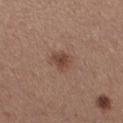Captured during whole-body skin photography for melanoma surveillance; the lesion was not biopsied.
A female subject, in their 30s.
The tile uses white-light illumination.
Approximately 2.5 mm at its widest.
On the right thigh.
This image is a 15 mm lesion crop taken from a total-body photograph.
Automated tile analysis of the lesion measured an area of roughly 5.5 mm², an outline eccentricity of about 0.45 (0 = round, 1 = elongated), and two-axis asymmetry of about 0.3.